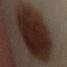Part of a total-body skin-imaging series; this lesion was reviewed on a skin check and was not flagged for biopsy. A male patient aged approximately 50. Automated image analysis of the tile measured a lesion color around L≈18 a*≈12 b*≈16 in CIELAB, roughly 12 lightness units darker than nearby skin, and a normalized border contrast of about 15. The analysis additionally found a classifier nevus-likeness of about 100/100. From the left lower leg. This image is a 15 mm lesion crop taken from a total-body photograph. Approximately 16 mm at its widest. The tile uses cross-polarized illumination.